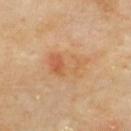This is a cross-polarized tile. The lesion is on the upper back. An algorithmic analysis of the crop reported a footprint of about 12 mm², an outline eccentricity of about 0.8 (0 = round, 1 = elongated), and two-axis asymmetry of about 0.3. The software also gave border irregularity of about 4 on a 0–10 scale, internal color variation of about 8.5 on a 0–10 scale, and radial color variation of about 3. And it measured a detector confidence of about 100 out of 100 that the crop contains a lesion. A male patient, aged 63 to 67. A roughly 15 mm field-of-view crop from a total-body skin photograph.